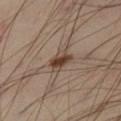workup: catalogued during a skin exam; not biopsied | patient: male, aged around 40 | imaging modality: ~15 mm tile from a whole-body skin photo | automated metrics: a footprint of about 5.5 mm² and a shape-asymmetry score of about 0.2 (0 = symmetric); a detector confidence of about 100 out of 100 that the crop contains a lesion | location: the right thigh | tile lighting: cross-polarized illumination | lesion size: about 3.5 mm.